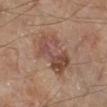{"biopsy_status": "not biopsied; imaged during a skin examination", "image": {"source": "total-body photography crop", "field_of_view_mm": 15}, "lighting": "cross-polarized", "patient": {"sex": "male", "age_approx": 65}, "lesion_size": {"long_diameter_mm_approx": 7.0}, "site": "leg"}